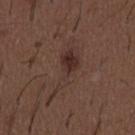Q: Was this lesion biopsied?
A: total-body-photography surveillance lesion; no biopsy
Q: What kind of image is this?
A: total-body-photography crop, ~15 mm field of view
Q: Patient demographics?
A: male, aged 48 to 52
Q: Automated lesion metrics?
A: an area of roughly 12 mm², an eccentricity of roughly 0.95, and a symmetry-axis asymmetry near 0.55; a lesion–skin lightness drop of about 6 and a normalized border contrast of about 6.5
Q: Lesion location?
A: the abdomen
Q: What lighting was used for the tile?
A: white-light
Q: What is the lesion's diameter?
A: ~8 mm (longest diameter)